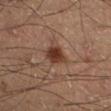Q: Was this lesion biopsied?
A: imaged on a skin check; not biopsied
Q: Illumination type?
A: cross-polarized illumination
Q: Who is the patient?
A: male, about 60 years old
Q: Where on the body is the lesion?
A: the right lower leg
Q: Lesion size?
A: about 3 mm
Q: What is the imaging modality?
A: ~15 mm crop, total-body skin-cancer survey